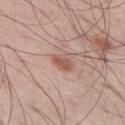This lesion was catalogued during total-body skin photography and was not selected for biopsy.
Automated tile analysis of the lesion measured a lesion area of about 4 mm², an eccentricity of roughly 0.8, and a shape-asymmetry score of about 0.2 (0 = symmetric). It also reported border irregularity of about 2 on a 0–10 scale and a within-lesion color-variation index near 1.5/10. The software also gave a nevus-likeness score of about 50/100 and a lesion-detection confidence of about 100/100.
From the left thigh.
Captured under white-light illumination.
The subject is a male approximately 60 years of age.
A close-up tile cropped from a whole-body skin photograph, about 15 mm across.